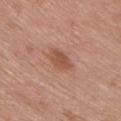Notes:
• follow-up — no biopsy performed (imaged during a skin exam)
• TBP lesion metrics — a symmetry-axis asymmetry near 0.2; a lesion color around L≈52 a*≈24 b*≈30 in CIELAB, roughly 9 lightness units darker than nearby skin, and a normalized border contrast of about 7; a border-irregularity rating of about 2/10, a color-variation rating of about 2/10, and radial color variation of about 0.5; a nevus-likeness score of about 60/100 and a detector confidence of about 100 out of 100 that the crop contains a lesion
• tile lighting — white-light
• image — total-body-photography crop, ~15 mm field of view
• site — the chest
• patient — male, aged 53–57
• lesion size — about 3.5 mm